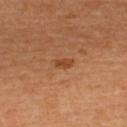This lesion was catalogued during total-body skin photography and was not selected for biopsy. A female subject, about 30 years old. Automated tile analysis of the lesion measured a lesion area of about 2.5 mm², an eccentricity of roughly 0.85, and a symmetry-axis asymmetry near 0.35. The analysis additionally found a lesion color around L≈46 a*≈25 b*≈39 in CIELAB. And it measured border irregularity of about 3 on a 0–10 scale, internal color variation of about 0 on a 0–10 scale, and radial color variation of about 0. Longest diameter approximately 2 mm. On the upper back. A 15 mm close-up tile from a total-body photography series done for melanoma screening. Captured under cross-polarized illumination.